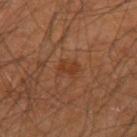Recorded during total-body skin imaging; not selected for excision or biopsy.
An algorithmic analysis of the crop reported a footprint of about 4 mm², an eccentricity of roughly 0.75, and a shape-asymmetry score of about 0.25 (0 = symmetric). It also reported a lesion color around L≈36 a*≈22 b*≈32 in CIELAB and a normalized lesion–skin contrast near 5.5. And it measured a border-irregularity rating of about 3/10, a color-variation rating of about 2/10, and a peripheral color-asymmetry measure near 1. The software also gave a nevus-likeness score of about 5/100 and a lesion-detection confidence of about 100/100.
The recorded lesion diameter is about 2.5 mm.
This is a cross-polarized tile.
A male patient in their mid- to late 60s.
A lesion tile, about 15 mm wide, cut from a 3D total-body photograph.
The lesion is located on the arm.